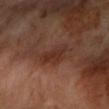Impression: Imaged during a routine full-body skin examination; the lesion was not biopsied and no histopathology is available. Image and clinical context: This is a cross-polarized tile. Cropped from a whole-body photographic skin survey; the tile spans about 15 mm. Located on the left forearm. The recorded lesion diameter is about 3.5 mm. A female subject, approximately 60 years of age.